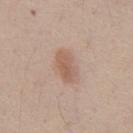– biopsy status — catalogued during a skin exam; not biopsied
– imaging modality — total-body-photography crop, ~15 mm field of view
– lesion size — ≈4 mm
– subject — male, aged 38 to 42
– site — the abdomen
– image-analysis metrics — an eccentricity of roughly 0.8 and two-axis asymmetry of about 0.15; a lesion color around L≈58 a*≈19 b*≈28 in CIELAB and about 9 CIELAB-L* units darker than the surrounding skin; a border-irregularity index near 1.5/10, internal color variation of about 4 on a 0–10 scale, and radial color variation of about 1.5; a classifier nevus-likeness of about 80/100 and a lesion-detection confidence of about 100/100